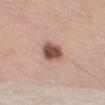{
  "biopsy_status": "not biopsied; imaged during a skin examination",
  "patient": {
    "sex": "male",
    "age_approx": 55
  },
  "image": {
    "source": "total-body photography crop",
    "field_of_view_mm": 15
  },
  "site": "left upper arm",
  "lighting": "white-light"
}